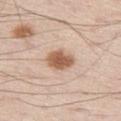Assessment: No biopsy was performed on this lesion — it was imaged during a full skin examination and was not determined to be concerning. Background: A 15 mm crop from a total-body photograph taken for skin-cancer surveillance. Located on the left thigh. A male subject aged 58–62.